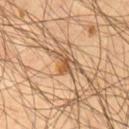• body site — the chest
• patient — male, roughly 55 years of age
• automated metrics — a lesion color around L≈52 a*≈21 b*≈37 in CIELAB, about 11 CIELAB-L* units darker than the surrounding skin, and a lesion-to-skin contrast of about 8.5 (normalized; higher = more distinct)
• tile lighting — cross-polarized
• image — total-body-photography crop, ~15 mm field of view